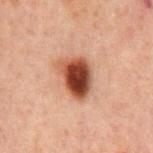Q: Was this lesion biopsied?
A: catalogued during a skin exam; not biopsied
Q: What is the lesion's diameter?
A: about 4.5 mm
Q: What did automated image analysis measure?
A: border irregularity of about 2.5 on a 0–10 scale and radial color variation of about 2.5
Q: What kind of image is this?
A: 15 mm crop, total-body photography
Q: Where on the body is the lesion?
A: the chest
Q: What are the patient's age and sex?
A: male, aged 58–62